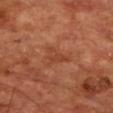Assessment:
Captured during whole-body skin photography for melanoma surveillance; the lesion was not biopsied.
Background:
A male subject in their 70s. From the chest. A 15 mm close-up tile from a total-body photography series done for melanoma screening. Captured under cross-polarized illumination.